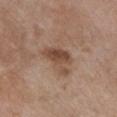follow-up = total-body-photography surveillance lesion; no biopsy | location = the front of the torso | imaging modality = ~15 mm crop, total-body skin-cancer survey | patient = female, in their mid-60s.